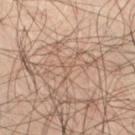lesion diameter: about 1.5 mm; imaging modality: ~15 mm crop, total-body skin-cancer survey; subject: male, in their mid-60s; location: the right thigh.